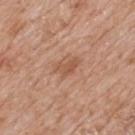Assessment:
Imaged during a routine full-body skin examination; the lesion was not biopsied and no histopathology is available.
Acquisition and patient details:
Captured under white-light illumination. A close-up tile cropped from a whole-body skin photograph, about 15 mm across. Approximately 3 mm at its widest. From the mid back. A male patient aged 58 to 62.